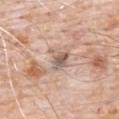workup: imaged on a skin check; not biopsied | automated lesion analysis: a footprint of about 5.5 mm², an eccentricity of roughly 0.5, and a shape-asymmetry score of about 0.5 (0 = symmetric); about 11 CIELAB-L* units darker than the surrounding skin; a border-irregularity rating of about 5.5/10 and peripheral color asymmetry of about 2; a classifier nevus-likeness of about 0/100 and a detector confidence of about 65 out of 100 that the crop contains a lesion | acquisition: ~15 mm tile from a whole-body skin photo | patient: male, approximately 80 years of age | diameter: ≈2.5 mm | tile lighting: white-light | body site: the chest.